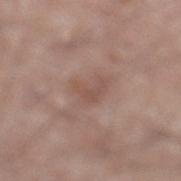Assessment: Imaged during a routine full-body skin examination; the lesion was not biopsied and no histopathology is available. Clinical summary: The patient is a male aged around 60. This is a white-light tile. Cropped from a whole-body photographic skin survey; the tile spans about 15 mm. Located on the left lower leg.